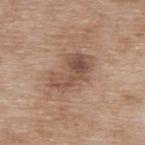Case summary:
* notes — catalogued during a skin exam; not biopsied
* TBP lesion metrics — about 10 CIELAB-L* units darker than the surrounding skin and a normalized lesion–skin contrast near 7; a nevus-likeness score of about 15/100 and lesion-presence confidence of about 100/100
* size — ≈5.5 mm
* tile lighting — white-light
* location — the upper back
* subject — female, aged approximately 75
* image source — ~15 mm crop, total-body skin-cancer survey